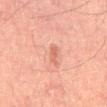notes: total-body-photography surveillance lesion; no biopsy
diameter: ≈2.5 mm
site: the mid back
lighting: cross-polarized
image source: total-body-photography crop, ~15 mm field of view
subject: male, in their mid- to late 40s
TBP lesion metrics: a nevus-likeness score of about 5/100